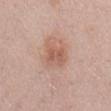Q: Was this lesion biopsied?
A: imaged on a skin check; not biopsied
Q: How was the tile lit?
A: white-light illumination
Q: Where on the body is the lesion?
A: the mid back
Q: Patient demographics?
A: male, about 55 years old
Q: What did automated image analysis measure?
A: an automated nevus-likeness rating near 25 out of 100
Q: What kind of image is this?
A: ~15 mm tile from a whole-body skin photo